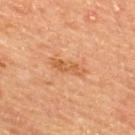Q: Was this lesion biopsied?
A: imaged on a skin check; not biopsied
Q: Where on the body is the lesion?
A: the upper back
Q: Who is the patient?
A: male, aged around 65
Q: Automated lesion metrics?
A: an area of roughly 5 mm² and a shape eccentricity near 0.95; a classifier nevus-likeness of about 0/100
Q: Illumination type?
A: cross-polarized
Q: Lesion size?
A: ≈4 mm
Q: How was this image acquired?
A: 15 mm crop, total-body photography